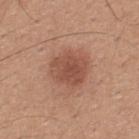notes: no biopsy performed (imaged during a skin exam); subject: male, aged approximately 30; acquisition: ~15 mm tile from a whole-body skin photo; location: the upper back.